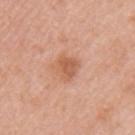Impression:
No biopsy was performed on this lesion — it was imaged during a full skin examination and was not determined to be concerning.
Background:
The subject is a female aged 38–42. Approximately 3 mm at its widest. A roughly 15 mm field-of-view crop from a total-body skin photograph. The tile uses white-light illumination. From the left upper arm.